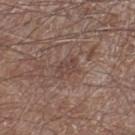The lesion was photographed on a routine skin check and not biopsied; there is no pathology result. A lesion tile, about 15 mm wide, cut from a 3D total-body photograph. A male patient aged 58 to 62. The lesion is on the right lower leg.